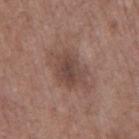  biopsy_status: not biopsied; imaged during a skin examination